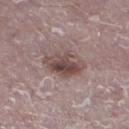Q: Was this lesion biopsied?
A: total-body-photography surveillance lesion; no biopsy
Q: What kind of image is this?
A: total-body-photography crop, ~15 mm field of view
Q: Who is the patient?
A: male, aged 73–77
Q: What did automated image analysis measure?
A: a classifier nevus-likeness of about 65/100 and lesion-presence confidence of about 100/100
Q: What is the anatomic site?
A: the left lower leg
Q: Lesion size?
A: ≈4.5 mm